The lesion is located on the upper back · a male patient, in their mid- to late 60s · a 15 mm crop from a total-body photograph taken for skin-cancer surveillance.
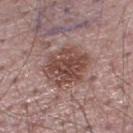About 5.5 mm across. Imaged with white-light lighting. The biopsy diagnosis was a dysplastic (Clark) nevus.